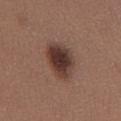Q: Was a biopsy performed?
A: imaged on a skin check; not biopsied
Q: Patient demographics?
A: female, aged approximately 55
Q: What kind of image is this?
A: ~15 mm tile from a whole-body skin photo
Q: Where on the body is the lesion?
A: the chest
Q: How large is the lesion?
A: ≈5 mm
Q: Illumination type?
A: white-light illumination
Q: What did automated image analysis measure?
A: a mean CIELAB color near L≈36 a*≈18 b*≈22 and a normalized border contrast of about 11.5